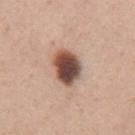The lesion was photographed on a routine skin check and not biopsied; there is no pathology result. A female subject aged approximately 30. A 15 mm close-up tile from a total-body photography series done for melanoma screening. The lesion is located on the chest.